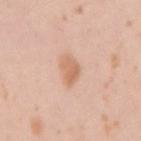Clinical impression: This lesion was catalogued during total-body skin photography and was not selected for biopsy. Acquisition and patient details: Imaged with white-light lighting. Approximately 3 mm at its widest. The subject is a female aged 18–22. Located on the right upper arm. A lesion tile, about 15 mm wide, cut from a 3D total-body photograph.